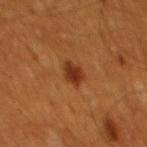biopsy status=catalogued during a skin exam; not biopsied | site=the back | subject=male, aged around 60 | acquisition=15 mm crop, total-body photography.